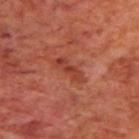Imaged during a routine full-body skin examination; the lesion was not biopsied and no histopathology is available. A male patient, aged approximately 70. Imaged with cross-polarized lighting. A 15 mm close-up tile from a total-body photography series done for melanoma screening. An algorithmic analysis of the crop reported an area of roughly 4 mm², an eccentricity of roughly 0.95, and a shape-asymmetry score of about 0.3 (0 = symmetric). It also reported a lesion color around L≈40 a*≈30 b*≈31 in CIELAB and a lesion-to-skin contrast of about 6.5 (normalized; higher = more distinct). The analysis additionally found border irregularity of about 4.5 on a 0–10 scale and a within-lesion color-variation index near 0/10. About 4 mm across. The lesion is located on the upper back.